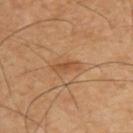site — the upper back
automated metrics — a footprint of about 3.5 mm², an outline eccentricity of about 0.95 (0 = round, 1 = elongated), and two-axis asymmetry of about 0.25; a mean CIELAB color near L≈49 a*≈21 b*≈36, a lesion–skin lightness drop of about 8, and a normalized lesion–skin contrast near 6; a border-irregularity index near 3.5/10
subject — male, aged around 60
acquisition — ~15 mm crop, total-body skin-cancer survey